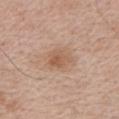The lesion was tiled from a total-body skin photograph and was not biopsied.
The patient is a male aged approximately 65.
A close-up tile cropped from a whole-body skin photograph, about 15 mm across.
The total-body-photography lesion software estimated a lesion area of about 5 mm² and a shape-asymmetry score of about 0.3 (0 = symmetric). The analysis additionally found a classifier nevus-likeness of about 30/100 and a detector confidence of about 100 out of 100 that the crop contains a lesion.
Approximately 3 mm at its widest.
Located on the chest.